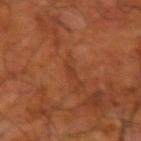Recorded during total-body skin imaging; not selected for excision or biopsy. A 15 mm close-up extracted from a 3D total-body photography capture. The lesion is located on the right upper arm. A male patient, aged 63–67.